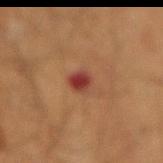Notes:
• notes: imaged on a skin check; not biopsied
• image source: total-body-photography crop, ~15 mm field of view
• anatomic site: the abdomen
• patient: male, aged 58–62
• lesion diameter: about 3 mm